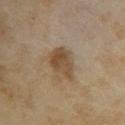Recorded during total-body skin imaging; not selected for excision or biopsy. The subject is a female aged around 35. A roughly 15 mm field-of-view crop from a total-body skin photograph. The tile uses cross-polarized illumination. The lesion is on the upper back.